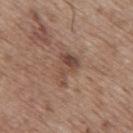{
  "biopsy_status": "not biopsied; imaged during a skin examination",
  "patient": {
    "sex": "male",
    "age_approx": 70
  },
  "site": "back",
  "automated_metrics": {
    "area_mm2_approx": 7.5,
    "eccentricity": 0.8,
    "shape_asymmetry": 0.5,
    "cielab_L": 47,
    "cielab_a": 18,
    "cielab_b": 26,
    "vs_skin_contrast_norm": 7.0,
    "border_irregularity_0_10": 5.0,
    "color_variation_0_10": 6.0,
    "peripheral_color_asymmetry": 2.0,
    "nevus_likeness_0_100": 10
  },
  "lighting": "white-light",
  "image": {
    "source": "total-body photography crop",
    "field_of_view_mm": 15
  },
  "lesion_size": {
    "long_diameter_mm_approx": 4.0
  }
}